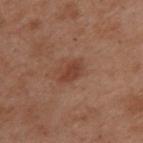Impression: This lesion was catalogued during total-body skin photography and was not selected for biopsy. Image and clinical context: A roughly 15 mm field-of-view crop from a total-body skin photograph. The total-body-photography lesion software estimated an average lesion color of about L≈33 a*≈19 b*≈24 (CIELAB). The analysis additionally found lesion-presence confidence of about 100/100. Located on the back. The patient is a female approximately 55 years of age. The lesion's longest dimension is about 3 mm. Captured under cross-polarized illumination.